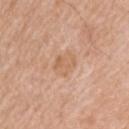Q: Was this lesion biopsied?
A: total-body-photography surveillance lesion; no biopsy
Q: What is the lesion's diameter?
A: about 3.5 mm
Q: Illumination type?
A: white-light
Q: What is the imaging modality?
A: total-body-photography crop, ~15 mm field of view
Q: Who is the patient?
A: male, approximately 80 years of age
Q: What is the anatomic site?
A: the upper back